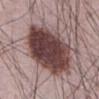Clinical impression: Imaged during a routine full-body skin examination; the lesion was not biopsied and no histopathology is available. Image and clinical context: Imaged with white-light lighting. The lesion is located on the front of the torso. The total-body-photography lesion software estimated an area of roughly 40 mm² and an outline eccentricity of about 0.7 (0 = round, 1 = elongated). And it measured an automated nevus-likeness rating near 95 out of 100 and lesion-presence confidence of about 100/100. A close-up tile cropped from a whole-body skin photograph, about 15 mm across. About 8.5 mm across. A male patient, in their mid-60s.